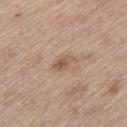{"biopsy_status": "not biopsied; imaged during a skin examination", "image": {"source": "total-body photography crop", "field_of_view_mm": 15}, "automated_metrics": {"cielab_L": 55, "cielab_a": 17, "cielab_b": 30, "vs_skin_contrast_norm": 7.0, "nevus_likeness_0_100": 5, "lesion_detection_confidence_0_100": 100}, "patient": {"sex": "male", "age_approx": 70}, "lesion_size": {"long_diameter_mm_approx": 3.0}, "site": "leg"}